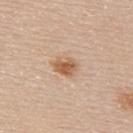Notes:
- notes — imaged on a skin check; not biopsied
- diameter — about 3 mm
- patient — male, in their 60s
- image source — ~15 mm crop, total-body skin-cancer survey
- anatomic site — the upper back
- lighting — white-light illumination